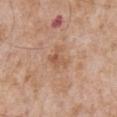{"biopsy_status": "not biopsied; imaged during a skin examination", "lesion_size": {"long_diameter_mm_approx": 3.0}, "lighting": "white-light", "patient": {"sex": "male", "age_approx": 65}, "image": {"source": "total-body photography crop", "field_of_view_mm": 15}, "site": "front of the torso"}